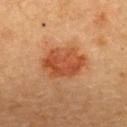Findings:
- follow-up · no biopsy performed (imaged during a skin exam)
- location · the upper back
- lighting · cross-polarized illumination
- subject · female, aged around 60
- TBP lesion metrics · a footprint of about 16 mm²; an average lesion color of about L≈45 a*≈26 b*≈35 (CIELAB) and a normalized border contrast of about 8
- image source · total-body-photography crop, ~15 mm field of view